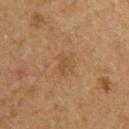A male patient, in their 60s. A 15 mm close-up tile from a total-body photography series done for melanoma screening.